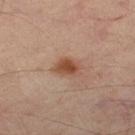Clinical impression: The lesion was photographed on a routine skin check and not biopsied; there is no pathology result. Image and clinical context: A 15 mm crop from a total-body photograph taken for skin-cancer surveillance. The patient is aged 53 to 57. The total-body-photography lesion software estimated an area of roughly 5.5 mm², an eccentricity of roughly 0.7, and a symmetry-axis asymmetry near 0.25. And it measured a border-irregularity index near 2.5/10, internal color variation of about 4 on a 0–10 scale, and radial color variation of about 1.5. Located on the right thigh.